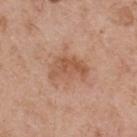Case summary:
* follow-up · no biopsy performed (imaged during a skin exam)
* lighting · white-light
* subject · male, aged around 55
* imaging modality · ~15 mm tile from a whole-body skin photo
* site · the upper back
* image-analysis metrics · an area of roughly 9.5 mm², a shape eccentricity near 0.8, and two-axis asymmetry of about 0.4; internal color variation of about 3.5 on a 0–10 scale and a peripheral color-asymmetry measure near 1.5; an automated nevus-likeness rating near 20 out of 100 and lesion-presence confidence of about 100/100
* size · ~4.5 mm (longest diameter)